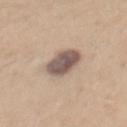Clinical impression: This lesion was catalogued during total-body skin photography and was not selected for biopsy. Image and clinical context: A male subject aged 33 to 37. Approximately 4.5 mm at its widest. The lesion is on the mid back. Automated tile analysis of the lesion measured a lesion color around L≈54 a*≈14 b*≈22 in CIELAB and about 16 CIELAB-L* units darker than the surrounding skin. Cropped from a whole-body photographic skin survey; the tile spans about 15 mm.